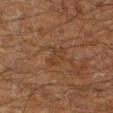{"biopsy_status": "not biopsied; imaged during a skin examination", "site": "left lower leg", "lesion_size": {"long_diameter_mm_approx": 3.0}, "patient": {"sex": "male", "age_approx": 60}, "image": {"source": "total-body photography crop", "field_of_view_mm": 15}, "lighting": "cross-polarized"}